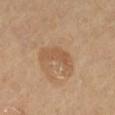No biopsy was performed on this lesion — it was imaged during a full skin examination and was not determined to be concerning. A subject roughly 65 years of age. Located on the left thigh. Longest diameter approximately 4.5 mm. This is a cross-polarized tile. Automated tile analysis of the lesion measured an area of roughly 8 mm² and a symmetry-axis asymmetry near 0.5. It also reported an average lesion color of about L≈53 a*≈18 b*≈33 (CIELAB), about 7 CIELAB-L* units darker than the surrounding skin, and a normalized lesion–skin contrast near 5.5. And it measured lesion-presence confidence of about 100/100. This image is a 15 mm lesion crop taken from a total-body photograph.